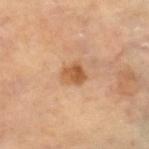Impression:
The lesion was tiled from a total-body skin photograph and was not biopsied.
Clinical summary:
A female subject in their mid- to late 60s. Captured under cross-polarized illumination. A 15 mm close-up tile from a total-body photography series done for melanoma screening. Automated image analysis of the tile measured a shape eccentricity near 0.5. It also reported a mean CIELAB color near L≈56 a*≈23 b*≈38, about 11 CIELAB-L* units darker than the surrounding skin, and a normalized lesion–skin contrast near 8. On the right lower leg.